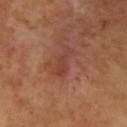Recorded during total-body skin imaging; not selected for excision or biopsy. The subject is a male roughly 65 years of age. A roughly 15 mm field-of-view crop from a total-body skin photograph. From the upper back. The lesion's longest dimension is about 3.5 mm. Imaged with cross-polarized lighting.